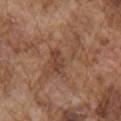- biopsy status — imaged on a skin check; not biopsied